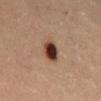follow-up: catalogued during a skin exam; not biopsied | anatomic site: the front of the torso | image: 15 mm crop, total-body photography | automated metrics: a lesion area of about 6.5 mm², an eccentricity of roughly 0.75, and a symmetry-axis asymmetry near 0.15 | size: ~3.5 mm (longest diameter) | subject: female, in their mid- to late 40s.